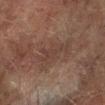Q: What are the patient's age and sex?
A: male, aged approximately 75
Q: How was the tile lit?
A: cross-polarized
Q: What kind of image is this?
A: ~15 mm tile from a whole-body skin photo
Q: Where on the body is the lesion?
A: the left lower leg